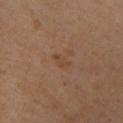workup: total-body-photography surveillance lesion; no biopsy | TBP lesion metrics: an area of roughly 2.5 mm², an outline eccentricity of about 0.85 (0 = round, 1 = elongated), and a symmetry-axis asymmetry near 0.45; a mean CIELAB color near L≈43 a*≈19 b*≈29, about 5 CIELAB-L* units darker than the surrounding skin, and a normalized lesion–skin contrast near 5; an automated nevus-likeness rating near 0 out of 100 | subject: male, about 50 years old | acquisition: ~15 mm crop, total-body skin-cancer survey | location: the upper back.